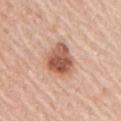follow-up=no biopsy performed (imaged during a skin exam)
lesion size=≈4 mm
anatomic site=the right upper arm
imaging modality=15 mm crop, total-body photography
subject=male, aged approximately 65